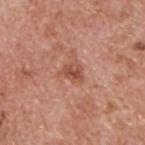Assessment: No biopsy was performed on this lesion — it was imaged during a full skin examination and was not determined to be concerning. Context: Automated image analysis of the tile measured a border-irregularity index near 3.5/10, internal color variation of about 3.5 on a 0–10 scale, and radial color variation of about 1.5. Cropped from a whole-body photographic skin survey; the tile spans about 15 mm. About 3 mm across. Located on the upper back. This is a white-light tile. A male patient aged approximately 60.